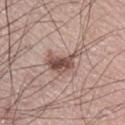{
  "biopsy_status": "not biopsied; imaged during a skin examination",
  "patient": {
    "sex": "male",
    "age_approx": 70
  },
  "site": "leg",
  "image": {
    "source": "total-body photography crop",
    "field_of_view_mm": 15
  }
}